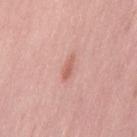biopsy status: imaged on a skin check; not biopsied
illumination: white-light illumination
patient: female, aged around 40
image source: ~15 mm crop, total-body skin-cancer survey
automated metrics: border irregularity of about 4.5 on a 0–10 scale and radial color variation of about 0
site: the leg